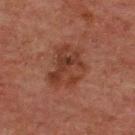{
  "biopsy_status": "not biopsied; imaged during a skin examination",
  "site": "upper back",
  "patient": {
    "sex": "male",
    "age_approx": 60
  },
  "automated_metrics": {
    "area_mm2_approx": 17.0,
    "shape_asymmetry": 0.2,
    "nevus_likeness_0_100": 5,
    "lesion_detection_confidence_0_100": 100
  },
  "lesion_size": {
    "long_diameter_mm_approx": 5.5
  },
  "image": {
    "source": "total-body photography crop",
    "field_of_view_mm": 15
  }
}